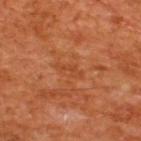<lesion>
  <biopsy_status>not biopsied; imaged during a skin examination</biopsy_status>
  <site>back</site>
  <image>
    <source>total-body photography crop</source>
    <field_of_view_mm>15</field_of_view_mm>
  </image>
  <patient>
    <sex>male</sex>
    <age_approx>65</age_approx>
  </patient>
</lesion>